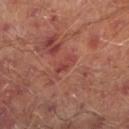Clinical impression: Captured during whole-body skin photography for melanoma surveillance; the lesion was not biopsied. Context: Imaged with cross-polarized lighting. Automated tile analysis of the lesion measured a lesion area of about 3 mm², a shape eccentricity near 0.9, and two-axis asymmetry of about 0.35. It also reported an average lesion color of about L≈42 a*≈29 b*≈25 (CIELAB) and a normalized lesion–skin contrast near 5.5. The software also gave a border-irregularity rating of about 4/10, a within-lesion color-variation index near 0/10, and peripheral color asymmetry of about 0. The software also gave a nevus-likeness score of about 0/100 and a detector confidence of about 100 out of 100 that the crop contains a lesion. Located on the left lower leg. Cropped from a total-body skin-imaging series; the visible field is about 15 mm. A male subject, in their mid- to late 60s. About 3 mm across.